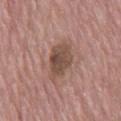{
  "biopsy_status": "not biopsied; imaged during a skin examination",
  "image": {
    "source": "total-body photography crop",
    "field_of_view_mm": 15
  },
  "site": "chest",
  "patient": {
    "sex": "male",
    "age_approx": 65
  },
  "lighting": "white-light",
  "automated_metrics": {
    "area_mm2_approx": 11.0,
    "eccentricity": 0.8,
    "shape_asymmetry": 0.25,
    "cielab_L": 49,
    "cielab_a": 18,
    "cielab_b": 25,
    "vs_skin_darker_L": 11.0,
    "vs_skin_contrast_norm": 8.0,
    "border_irregularity_0_10": 2.5,
    "peripheral_color_asymmetry": 1.0,
    "nevus_likeness_0_100": 30,
    "lesion_detection_confidence_0_100": 100
  },
  "lesion_size": {
    "long_diameter_mm_approx": 5.0
  }
}